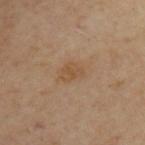  biopsy_status: not biopsied; imaged during a skin examination
  patient:
    age_approx: 55
  lesion_size:
    long_diameter_mm_approx: 4.0
  image:
    source: total-body photography crop
    field_of_view_mm: 15
  site: upper back
  lighting: cross-polarized